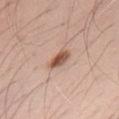notes — imaged on a skin check; not biopsied | image-analysis metrics — an area of roughly 4 mm², a shape eccentricity near 0.8, and two-axis asymmetry of about 0.2; a mean CIELAB color near L≈54 a*≈21 b*≈29 and a normalized border contrast of about 10; a border-irregularity rating of about 2/10, internal color variation of about 5 on a 0–10 scale, and a peripheral color-asymmetry measure near 2 | image — total-body-photography crop, ~15 mm field of view | illumination — white-light illumination | lesion diameter — ~3 mm (longest diameter) | patient — male, roughly 70 years of age | body site — the back.